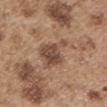This lesion was catalogued during total-body skin photography and was not selected for biopsy.
The tile uses white-light illumination.
Located on the left upper arm.
The patient is a male approximately 55 years of age.
Cropped from a whole-body photographic skin survey; the tile spans about 15 mm.